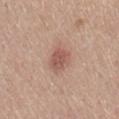Findings:
• notes · catalogued during a skin exam; not biopsied
• anatomic site · the mid back
• subject · male, approximately 60 years of age
• image source · ~15 mm tile from a whole-body skin photo